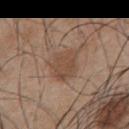Findings:
– biopsy status — catalogued during a skin exam; not biopsied
– size — about 4 mm
– acquisition — 15 mm crop, total-body photography
– patient — male, aged approximately 45
– location — the front of the torso
– illumination — white-light